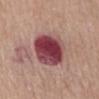location: the abdomen; acquisition: 15 mm crop, total-body photography; patient: male, roughly 80 years of age.